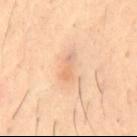This lesion was catalogued during total-body skin photography and was not selected for biopsy.
From the back.
Imaged with cross-polarized lighting.
A lesion tile, about 15 mm wide, cut from a 3D total-body photograph.
A male patient about 40 years old.
An algorithmic analysis of the crop reported an area of roughly 3.5 mm², an eccentricity of roughly 0.95, and a shape-asymmetry score of about 0.4 (0 = symmetric). The software also gave a mean CIELAB color near L≈70 a*≈21 b*≈36 and a lesion-to-skin contrast of about 5 (normalized; higher = more distinct). And it measured a border-irregularity index near 5/10, a color-variation rating of about 0.5/10, and radial color variation of about 0.
The lesion's longest dimension is about 3.5 mm.